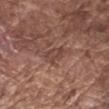| key | value |
|---|---|
| biopsy status | imaged on a skin check; not biopsied |
| anatomic site | the arm |
| patient | male, about 75 years old |
| image | 15 mm crop, total-body photography |
| lesion diameter | ≈3 mm |
| illumination | white-light illumination |